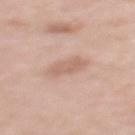biopsy status — imaged on a skin check; not biopsied
anatomic site — the upper back
lesion diameter — ~3.5 mm (longest diameter)
acquisition — total-body-photography crop, ~15 mm field of view
automated metrics — an area of roughly 6 mm²; a border-irregularity rating of about 2/10, a color-variation rating of about 1.5/10, and radial color variation of about 0.5; an automated nevus-likeness rating near 15 out of 100 and lesion-presence confidence of about 100/100
patient — female, approximately 40 years of age